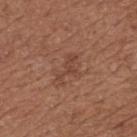Clinical impression: The lesion was photographed on a routine skin check and not biopsied; there is no pathology result. Context: The subject is a female aged around 65. This is a white-light tile. The lesion is located on the upper back. An algorithmic analysis of the crop reported a lesion area of about 4.5 mm², an eccentricity of roughly 0.9, and two-axis asymmetry of about 0.55. And it measured a nevus-likeness score of about 0/100. Cropped from a whole-body photographic skin survey; the tile spans about 15 mm.